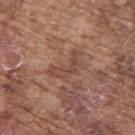Imaged during a routine full-body skin examination; the lesion was not biopsied and no histopathology is available. A close-up tile cropped from a whole-body skin photograph, about 15 mm across. Imaged with white-light lighting. Located on the upper back. The subject is a male aged approximately 75.